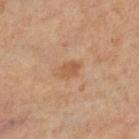| feature | finding |
|---|---|
| notes | total-body-photography surveillance lesion; no biopsy |
| patient | female, aged 63–67 |
| body site | the right leg |
| imaging modality | 15 mm crop, total-body photography |